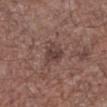workup: no biopsy performed (imaged during a skin exam) | size: ≈3 mm | image: total-body-photography crop, ~15 mm field of view | site: the front of the torso | patient: male, roughly 70 years of age | lighting: white-light | TBP lesion metrics: a lesion area of about 5 mm², an eccentricity of roughly 0.75, and two-axis asymmetry of about 0.3; a border-irregularity rating of about 3/10 and internal color variation of about 3 on a 0–10 scale.